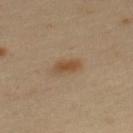Q: Was a biopsy performed?
A: no biopsy performed (imaged during a skin exam)